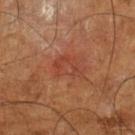Part of a total-body skin-imaging series; this lesion was reviewed on a skin check and was not flagged for biopsy. A roughly 15 mm field-of-view crop from a total-body skin photograph. A male subject, about 65 years old. The tile uses cross-polarized illumination. The lesion is located on the left lower leg. Automated image analysis of the tile measured a shape eccentricity near 0.75 and a shape-asymmetry score of about 0.3 (0 = symmetric). The software also gave about 6 CIELAB-L* units darker than the surrounding skin and a normalized border contrast of about 4.5. The analysis additionally found a classifier nevus-likeness of about 0/100 and a lesion-detection confidence of about 100/100. About 3.5 mm across.